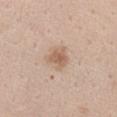The patient is a female about 35 years old. The lesion is located on the chest. The total-body-photography lesion software estimated an area of roughly 5.5 mm², an outline eccentricity of about 0.5 (0 = round, 1 = elongated), and a shape-asymmetry score of about 0.35 (0 = symmetric). The software also gave border irregularity of about 3.5 on a 0–10 scale, a within-lesion color-variation index near 2.5/10, and radial color variation of about 1. The analysis additionally found a nevus-likeness score of about 65/100 and a detector confidence of about 100 out of 100 that the crop contains a lesion. Approximately 3 mm at its widest. A lesion tile, about 15 mm wide, cut from a 3D total-body photograph.